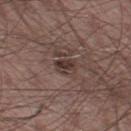Notes:
- workup · no biopsy performed (imaged during a skin exam)
- acquisition · total-body-photography crop, ~15 mm field of view
- body site · the leg
- lesion size · about 2.5 mm
- patient · male, roughly 65 years of age